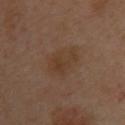| key | value |
|---|---|
| workup | imaged on a skin check; not biopsied |
| illumination | cross-polarized |
| lesion size | about 5 mm |
| site | the upper back |
| image source | ~15 mm crop, total-body skin-cancer survey |
| patient | female, aged 38–42 |